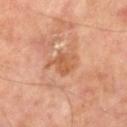Case summary:
– notes — total-body-photography surveillance lesion; no biopsy
– imaging modality — ~15 mm crop, total-body skin-cancer survey
– size — ≈3.5 mm
– location — the left leg
– patient — male, about 50 years old
– tile lighting — cross-polarized illumination